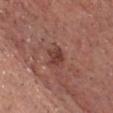  biopsy_status: not biopsied; imaged during a skin examination
  lighting: white-light
  image:
    source: total-body photography crop
    field_of_view_mm: 15
  lesion_size:
    long_diameter_mm_approx: 2.5
  patient:
    sex: male
    age_approx: 65
  site: head or neck